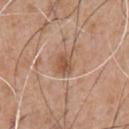<record>
  <biopsy_status>not biopsied; imaged during a skin examination</biopsy_status>
  <lesion_size>
    <long_diameter_mm_approx>2.5</long_diameter_mm_approx>
  </lesion_size>
  <site>chest</site>
  <image>
    <source>total-body photography crop</source>
    <field_of_view_mm>15</field_of_view_mm>
  </image>
  <patient>
    <sex>male</sex>
    <age_approx>65</age_approx>
  </patient>
</record>